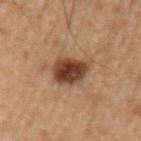biopsy status = imaged on a skin check; not biopsied
site = the arm
image = total-body-photography crop, ~15 mm field of view
illumination = cross-polarized illumination
subject = male, in their 70s
lesion size = ≈5 mm